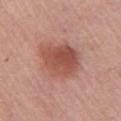Notes:
- follow-up · imaged on a skin check; not biopsied
- diameter · ~5.5 mm (longest diameter)
- subject · female, aged approximately 65
- automated metrics · a lesion area of about 17 mm², an eccentricity of roughly 0.65, and a shape-asymmetry score of about 0.25 (0 = symmetric); a mean CIELAB color near L≈51 a*≈26 b*≈28, a lesion–skin lightness drop of about 11, and a normalized border contrast of about 8
- image source · total-body-photography crop, ~15 mm field of view
- illumination · white-light
- body site · the right upper arm